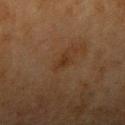Automated tile analysis of the lesion measured a symmetry-axis asymmetry near 0.35. It also reported roughly 4 lightness units darker than nearby skin. The analysis additionally found a border-irregularity index near 3.5/10, a within-lesion color-variation index near 1/10, and radial color variation of about 0.5. A region of skin cropped from a whole-body photographic capture, roughly 15 mm wide. The subject is a female in their mid-50s. Captured under cross-polarized illumination. Approximately 3 mm at its widest. The lesion is located on the right upper arm.